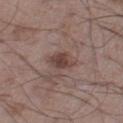Background:
The lesion is on the leg. Measured at roughly 3.5 mm in maximum diameter. A male patient aged approximately 50. Cropped from a total-body skin-imaging series; the visible field is about 15 mm. The tile uses white-light illumination.